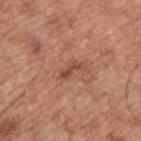Context: Captured under white-light illumination. Located on the left upper arm. A 15 mm close-up tile from a total-body photography series done for melanoma screening. Longest diameter approximately 2.5 mm. A male subject, in their mid- to late 50s. The total-body-photography lesion software estimated a lesion color around L≈48 a*≈24 b*≈31 in CIELAB. The software also gave a border-irregularity rating of about 4/10, internal color variation of about 0 on a 0–10 scale, and radial color variation of about 0. The analysis additionally found an automated nevus-likeness rating near 0 out of 100 and a lesion-detection confidence of about 100/100.